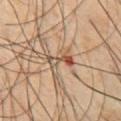biopsy status — no biopsy performed (imaged during a skin exam); size — ≈3.5 mm; body site — the chest; acquisition — ~15 mm tile from a whole-body skin photo; patient — male, aged 43 to 47; lighting — cross-polarized.